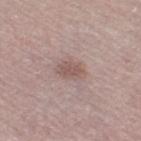Part of a total-body skin-imaging series; this lesion was reviewed on a skin check and was not flagged for biopsy.
Located on the right thigh.
Captured under white-light illumination.
A 15 mm close-up tile from a total-body photography series done for melanoma screening.
Longest diameter approximately 3 mm.
A female subject, roughly 65 years of age.
Automated image analysis of the tile measured a lesion area of about 5.5 mm², an eccentricity of roughly 0.7, and a symmetry-axis asymmetry near 0.2. The analysis additionally found a detector confidence of about 100 out of 100 that the crop contains a lesion.